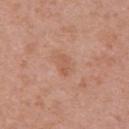No biopsy was performed on this lesion — it was imaged during a full skin examination and was not determined to be concerning. The tile uses white-light illumination. A female patient aged 38–42. A 15 mm close-up extracted from a 3D total-body photography capture. About 2.5 mm across. The lesion is located on the upper back. The lesion-visualizer software estimated an area of roughly 3 mm², an outline eccentricity of about 0.85 (0 = round, 1 = elongated), and a shape-asymmetry score of about 0.5 (0 = symmetric). It also reported a mean CIELAB color near L≈57 a*≈23 b*≈32, roughly 7 lightness units darker than nearby skin, and a lesion-to-skin contrast of about 5 (normalized; higher = more distinct). The analysis additionally found border irregularity of about 4.5 on a 0–10 scale, internal color variation of about 1 on a 0–10 scale, and a peripheral color-asymmetry measure near 0. It also reported a nevus-likeness score of about 0/100 and a lesion-detection confidence of about 100/100.